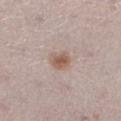This lesion was catalogued during total-body skin photography and was not selected for biopsy. The subject is a female aged approximately 20. The lesion is on the left lower leg. Captured under white-light illumination. A roughly 15 mm field-of-view crop from a total-body skin photograph. Automated image analysis of the tile measured an area of roughly 5 mm² and a shape eccentricity near 0.6. The analysis additionally found a lesion color around L≈57 a*≈17 b*≈26 in CIELAB, about 10 CIELAB-L* units darker than the surrounding skin, and a normalized border contrast of about 8.